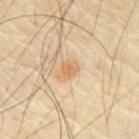The lesion was photographed on a routine skin check and not biopsied; there is no pathology result.
About 2.5 mm across.
Imaged with cross-polarized lighting.
The subject is a male aged around 65.
A 15 mm crop from a total-body photograph taken for skin-cancer surveillance.
Located on the chest.